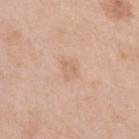Assessment:
Part of a total-body skin-imaging series; this lesion was reviewed on a skin check and was not flagged for biopsy.
Background:
Longest diameter approximately 2.5 mm. The patient is a female about 40 years old. The lesion-visualizer software estimated a footprint of about 4 mm², an eccentricity of roughly 0.6, and a symmetry-axis asymmetry near 0.25. And it measured border irregularity of about 2.5 on a 0–10 scale, internal color variation of about 2 on a 0–10 scale, and peripheral color asymmetry of about 1. And it measured a nevus-likeness score of about 0/100 and a lesion-detection confidence of about 100/100. This is a white-light tile. A 15 mm close-up extracted from a 3D total-body photography capture. The lesion is on the left upper arm.